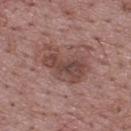<record>
  <biopsy_status>not biopsied; imaged during a skin examination</biopsy_status>
  <site>upper back</site>
  <lighting>white-light</lighting>
  <patient>
    <sex>male</sex>
    <age_approx>70</age_approx>
  </patient>
  <image>
    <source>total-body photography crop</source>
    <field_of_view_mm>15</field_of_view_mm>
  </image>
  <automated_metrics>
    <area_mm2_approx>15.0</area_mm2_approx>
    <eccentricity>0.85</eccentricity>
    <shape_asymmetry>0.4</shape_asymmetry>
    <border_irregularity_0_10>5.0</border_irregularity_0_10>
    <color_variation_0_10>4.0</color_variation_0_10>
    <peripheral_color_asymmetry>1.5</peripheral_color_asymmetry>
    <nevus_likeness_0_100>15</nevus_likeness_0_100>
  </automated_metrics>
</record>